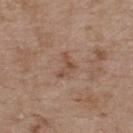Q: Is there a histopathology result?
A: catalogued during a skin exam; not biopsied
Q: Lesion location?
A: the upper back
Q: Patient demographics?
A: male, about 50 years old
Q: What did automated image analysis measure?
A: an area of roughly 3.5 mm², a shape eccentricity near 0.75, and two-axis asymmetry of about 0.65; a lesion color around L≈49 a*≈19 b*≈28 in CIELAB and a lesion–skin lightness drop of about 7; border irregularity of about 6.5 on a 0–10 scale, a within-lesion color-variation index near 0/10, and peripheral color asymmetry of about 0
Q: How was this image acquired?
A: ~15 mm crop, total-body skin-cancer survey
Q: What lighting was used for the tile?
A: white-light